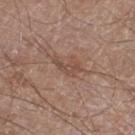Impression: This lesion was catalogued during total-body skin photography and was not selected for biopsy. Clinical summary: From the left lower leg. This is a white-light tile. A 15 mm crop from a total-body photograph taken for skin-cancer surveillance. The lesion-visualizer software estimated a shape-asymmetry score of about 0.5 (0 = symmetric). The software also gave a mean CIELAB color near L≈48 a*≈18 b*≈26 and a lesion–skin lightness drop of about 7. The software also gave border irregularity of about 5.5 on a 0–10 scale, a color-variation rating of about 1.5/10, and radial color variation of about 0.5. The lesion's longest dimension is about 3 mm. The patient is a male aged 58 to 62.